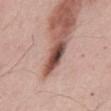About 4 mm across.
A male patient, aged approximately 55.
From the back.
Imaged with white-light lighting.
Cropped from a total-body skin-imaging series; the visible field is about 15 mm.